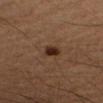Impression:
No biopsy was performed on this lesion — it was imaged during a full skin examination and was not determined to be concerning.
Acquisition and patient details:
A female patient aged around 55. Captured under cross-polarized illumination. The lesion-visualizer software estimated a mean CIELAB color near L≈26 a*≈18 b*≈25. On the right forearm. The recorded lesion diameter is about 2 mm. A 15 mm close-up extracted from a 3D total-body photography capture.